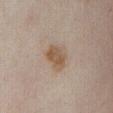– biopsy status · total-body-photography surveillance lesion; no biopsy
– image-analysis metrics · an eccentricity of roughly 0.75 and two-axis asymmetry of about 0.25
– patient · female, aged 33–37
– lesion diameter · ≈3.5 mm
– site · the abdomen
– image · ~15 mm crop, total-body skin-cancer survey